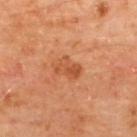An algorithmic analysis of the crop reported an area of roughly 5 mm². The software also gave about 8 CIELAB-L* units darker than the surrounding skin and a normalized lesion–skin contrast near 6.5. It also reported a nevus-likeness score of about 15/100 and a lesion-detection confidence of about 100/100. A male patient about 65 years old. A 15 mm close-up extracted from a 3D total-body photography capture. The lesion is located on the upper back. The tile uses cross-polarized illumination. The lesion's longest dimension is about 3 mm.